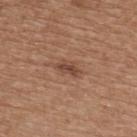Q: What is the anatomic site?
A: the upper back
Q: What lighting was used for the tile?
A: white-light illumination
Q: What are the patient's age and sex?
A: female, about 75 years old
Q: What is the imaging modality?
A: ~15 mm crop, total-body skin-cancer survey